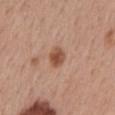Clinical impression:
Imaged during a routine full-body skin examination; the lesion was not biopsied and no histopathology is available.
Context:
From the mid back. The lesion's longest dimension is about 2.5 mm. A close-up tile cropped from a whole-body skin photograph, about 15 mm across. A female subject roughly 55 years of age.